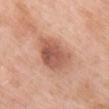biopsy status: catalogued during a skin exam; not biopsied | illumination: white-light illumination | location: the upper back | size: ~5.5 mm (longest diameter) | image: 15 mm crop, total-body photography | subject: female, aged 48 to 52.